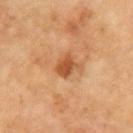Part of a total-body skin-imaging series; this lesion was reviewed on a skin check and was not flagged for biopsy. A male patient, aged approximately 70. The lesion is located on the arm. Longest diameter approximately 2.5 mm. A roughly 15 mm field-of-view crop from a total-body skin photograph. The total-body-photography lesion software estimated an average lesion color of about L≈52 a*≈26 b*≈40 (CIELAB) and a normalized lesion–skin contrast near 8. The analysis additionally found border irregularity of about 3 on a 0–10 scale, a within-lesion color-variation index near 2.5/10, and radial color variation of about 1. The analysis additionally found a nevus-likeness score of about 70/100 and lesion-presence confidence of about 100/100. The tile uses cross-polarized illumination.